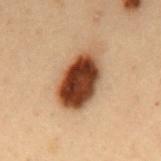<record>
<biopsy_status>not biopsied; imaged during a skin examination</biopsy_status>
</record>